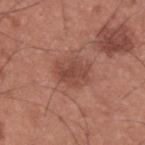Notes:
* biopsy status — total-body-photography surveillance lesion; no biopsy
* anatomic site — the upper back
* lighting — white-light
* patient — male, in their mid- to late 30s
* size — ~4 mm (longest diameter)
* image source — total-body-photography crop, ~15 mm field of view